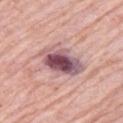Clinical impression: The lesion was tiled from a total-body skin photograph and was not biopsied. Context: The subject is a male aged 78 to 82. The lesion's longest dimension is about 5 mm. The lesion is located on the left upper arm. The tile uses white-light illumination. A 15 mm close-up extracted from a 3D total-body photography capture.